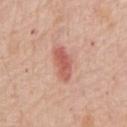notes — no biopsy performed (imaged during a skin exam) | location — the chest | subject — male, aged approximately 80 | imaging modality — 15 mm crop, total-body photography | automated metrics — an average lesion color of about L≈58 a*≈28 b*≈29 (CIELAB), a lesion–skin lightness drop of about 12, and a normalized border contrast of about 7.5; a nevus-likeness score of about 65/100 and a lesion-detection confidence of about 100/100 | illumination — white-light illumination | lesion size — about 4 mm.